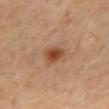This lesion was catalogued during total-body skin photography and was not selected for biopsy.
On the mid back.
The subject is a male aged around 55.
About 3 mm across.
A roughly 15 mm field-of-view crop from a total-body skin photograph.